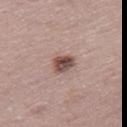No biopsy was performed on this lesion — it was imaged during a full skin examination and was not determined to be concerning. A roughly 15 mm field-of-view crop from a total-body skin photograph. Located on the right thigh. The total-body-photography lesion software estimated a mean CIELAB color near L≈49 a*≈18 b*≈22. And it measured a lesion-detection confidence of about 100/100. A female subject roughly 50 years of age.